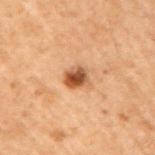workup: imaged on a skin check; not biopsied | imaging modality: ~15 mm tile from a whole-body skin photo | patient: male, about 70 years old | lighting: cross-polarized | anatomic site: the arm.